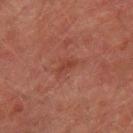* workup · total-body-photography surveillance lesion; no biopsy
* lesion size · about 3 mm
* patient · male, approximately 75 years of age
* image source · ~15 mm tile from a whole-body skin photo
* body site · the right thigh
* lighting · cross-polarized illumination